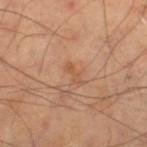workup: imaged on a skin check; not biopsied | acquisition: total-body-photography crop, ~15 mm field of view | patient: male, roughly 70 years of age | anatomic site: the left thigh.